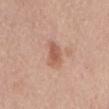Impression: Recorded during total-body skin imaging; not selected for excision or biopsy. Acquisition and patient details: The patient is a male in their mid-60s. Automated image analysis of the tile measured an area of roughly 7 mm² and an outline eccentricity of about 0.75 (0 = round, 1 = elongated). The software also gave a border-irregularity rating of about 3/10, a color-variation rating of about 3/10, and radial color variation of about 1. It also reported a nevus-likeness score of about 30/100 and a lesion-detection confidence of about 100/100. The lesion is located on the mid back. A 15 mm close-up tile from a total-body photography series done for melanoma screening. This is a white-light tile.